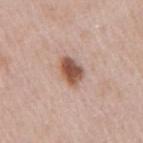Imaged during a routine full-body skin examination; the lesion was not biopsied and no histopathology is available. The lesion is on the arm. A region of skin cropped from a whole-body photographic capture, roughly 15 mm wide. A female subject, in their 40s.